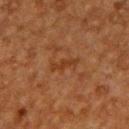workup: catalogued during a skin exam; not biopsied
lesion diameter: about 3 mm
site: the back
tile lighting: cross-polarized illumination
image source: total-body-photography crop, ~15 mm field of view
patient: male, aged 48 to 52
TBP lesion metrics: an average lesion color of about L≈30 a*≈20 b*≈30 (CIELAB) and a lesion-to-skin contrast of about 6.5 (normalized; higher = more distinct); an automated nevus-likeness rating near 0 out of 100